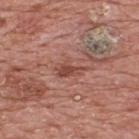| feature | finding |
|---|---|
| follow-up | total-body-photography surveillance lesion; no biopsy |
| image source | 15 mm crop, total-body photography |
| patient | male, approximately 70 years of age |
| site | the upper back |